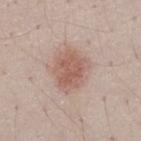Assessment: The lesion was tiled from a total-body skin photograph and was not biopsied. Clinical summary: This is a white-light tile. The lesion-visualizer software estimated a lesion area of about 15 mm², an outline eccentricity of about 0.6 (0 = round, 1 = elongated), and a symmetry-axis asymmetry near 0.2. The analysis additionally found a mean CIELAB color near L≈58 a*≈19 b*≈25, about 10 CIELAB-L* units darker than the surrounding skin, and a lesion-to-skin contrast of about 7 (normalized; higher = more distinct). The analysis additionally found border irregularity of about 2 on a 0–10 scale, internal color variation of about 3.5 on a 0–10 scale, and radial color variation of about 1. On the front of the torso. This image is a 15 mm lesion crop taken from a total-body photograph. A male subject, approximately 50 years of age. Longest diameter approximately 5 mm.